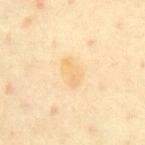  site: front of the torso
  image:
    source: total-body photography crop
    field_of_view_mm: 15
  patient:
    sex: male
    age_approx: 65